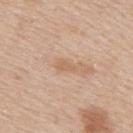Clinical impression: Recorded during total-body skin imaging; not selected for excision or biopsy. Acquisition and patient details: A male patient aged approximately 60. A lesion tile, about 15 mm wide, cut from a 3D total-body photograph. The lesion is located on the upper back.